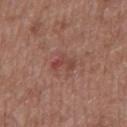| feature | finding |
|---|---|
| body site | the back |
| lesion diameter | about 3 mm |
| lighting | white-light |
| subject | male, aged 48 to 52 |
| automated lesion analysis | an average lesion color of about L≈44 a*≈25 b*≈25 (CIELAB), roughly 7 lightness units darker than nearby skin, and a lesion-to-skin contrast of about 6 (normalized; higher = more distinct) |
| image | 15 mm crop, total-body photography |